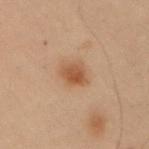Q: Is there a histopathology result?
A: imaged on a skin check; not biopsied
Q: What is the lesion's diameter?
A: ≈3.5 mm
Q: What are the patient's age and sex?
A: male, about 55 years old
Q: What kind of image is this?
A: 15 mm crop, total-body photography
Q: Automated lesion metrics?
A: a mean CIELAB color near L≈42 a*≈18 b*≈29, a lesion–skin lightness drop of about 9, and a normalized border contrast of about 8; a border-irregularity index near 2/10, a color-variation rating of about 3/10, and peripheral color asymmetry of about 1; an automated nevus-likeness rating near 100 out of 100
Q: What is the anatomic site?
A: the right upper arm